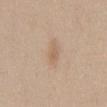  biopsy_status: not biopsied; imaged during a skin examination
  patient:
    sex: female
    age_approx: 40
  lighting: white-light
  lesion_size:
    long_diameter_mm_approx: 3.0
  image:
    source: total-body photography crop
    field_of_view_mm: 15
  automated_metrics:
    area_mm2_approx: 3.5
    eccentricity: 0.9
    shape_asymmetry: 0.25
    border_irregularity_0_10: 2.5
    color_variation_0_10: 1.0
    peripheral_color_asymmetry: 0.0
  site: back